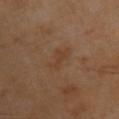{
  "automated_metrics": {
    "area_mm2_approx": 4.5,
    "cielab_L": 38,
    "cielab_a": 17,
    "cielab_b": 29,
    "vs_skin_darker_L": 4.0,
    "vs_skin_contrast_norm": 5.0,
    "color_variation_0_10": 1.0,
    "peripheral_color_asymmetry": 0.0,
    "nevus_likeness_0_100": 0,
    "lesion_detection_confidence_0_100": 100
  },
  "image": {
    "source": "total-body photography crop",
    "field_of_view_mm": 15
  },
  "lesion_size": {
    "long_diameter_mm_approx": 4.0
  },
  "patient": {
    "sex": "male",
    "age_approx": 70
  },
  "site": "upper back",
  "lighting": "cross-polarized"
}